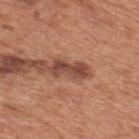Notes:
* biopsy status: imaged on a skin check; not biopsied
* tile lighting: white-light illumination
* automated metrics: a lesion area of about 6.5 mm² and two-axis asymmetry of about 0.3
* lesion diameter: ~4.5 mm (longest diameter)
* acquisition: 15 mm crop, total-body photography
* anatomic site: the upper back
* patient: male, in their mid-60s